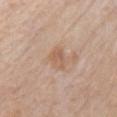This lesion was catalogued during total-body skin photography and was not selected for biopsy.
A male subject in their mid- to late 80s or older.
A 15 mm crop from a total-body photograph taken for skin-cancer surveillance.
The lesion is located on the chest.
Measured at roughly 3 mm in maximum diameter.
Imaged with white-light lighting.